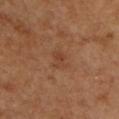• body site: the chest
• image: ~15 mm tile from a whole-body skin photo
• illumination: cross-polarized illumination
• lesion size: about 2.5 mm
• automated lesion analysis: a footprint of about 3.5 mm², an eccentricity of roughly 0.75, and two-axis asymmetry of about 0.3; an average lesion color of about L≈41 a*≈21 b*≈32 (CIELAB) and a normalized border contrast of about 5; border irregularity of about 3.5 on a 0–10 scale and a color-variation rating of about 1.5/10
• subject: male, about 50 years old